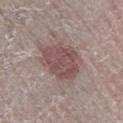This lesion was catalogued during total-body skin photography and was not selected for biopsy. A close-up tile cropped from a whole-body skin photograph, about 15 mm across. A male subject approximately 65 years of age. On the right lower leg.